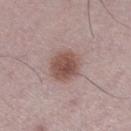follow-up: catalogued during a skin exam; not biopsied | subject: male, aged 48 to 52 | site: the leg | lesion diameter: about 4 mm | acquisition: 15 mm crop, total-body photography.